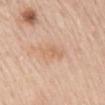follow-up = no biopsy performed (imaged during a skin exam)
image-analysis metrics = a mean CIELAB color near L≈66 a*≈19 b*≈34, about 6 CIELAB-L* units darker than the surrounding skin, and a normalized lesion–skin contrast near 5; a lesion-detection confidence of about 100/100
image = 15 mm crop, total-body photography
patient = female, about 70 years old
illumination = white-light illumination
location = the mid back